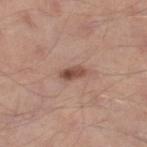{"biopsy_status": "not biopsied; imaged during a skin examination", "site": "right lower leg", "patient": {"sex": "male", "age_approx": 35}, "image": {"source": "total-body photography crop", "field_of_view_mm": 15}}